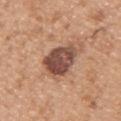The lesion was tiled from a total-body skin photograph and was not biopsied. A male patient aged approximately 30. The recorded lesion diameter is about 5 mm. A 15 mm close-up tile from a total-body photography series done for melanoma screening. From the right upper arm.